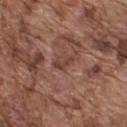This lesion was catalogued during total-body skin photography and was not selected for biopsy. Located on the upper back. A 15 mm close-up tile from a total-body photography series done for melanoma screening. Imaged with white-light lighting. The recorded lesion diameter is about 3 mm. The subject is a male roughly 75 years of age.